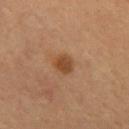No biopsy was performed on this lesion — it was imaged during a full skin examination and was not determined to be concerning. The total-body-photography lesion software estimated an average lesion color of about L≈41 a*≈20 b*≈32 (CIELAB), roughly 9 lightness units darker than nearby skin, and a lesion-to-skin contrast of about 8.5 (normalized; higher = more distinct). The software also gave lesion-presence confidence of about 100/100. A male patient, aged 53 to 57. A 15 mm crop from a total-body photograph taken for skin-cancer surveillance. On the back. This is a cross-polarized tile.